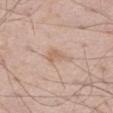  biopsy_status: not biopsied; imaged during a skin examination
  image:
    source: total-body photography crop
    field_of_view_mm: 15
  site: right thigh
  patient:
    sex: male
    age_approx: 55
  lighting: white-light